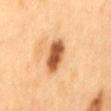A male patient aged 58 to 62. About 5 mm across. Captured under cross-polarized illumination. The lesion is on the mid back. A close-up tile cropped from a whole-body skin photograph, about 15 mm across.